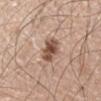notes = imaged on a skin check; not biopsied
diameter = ≈3 mm
site = the left forearm
automated lesion analysis = a border-irregularity index near 2/10; a lesion-detection confidence of about 100/100
patient = male, aged 58 to 62
lighting = white-light illumination
image = 15 mm crop, total-body photography